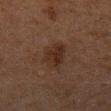No biopsy was performed on this lesion — it was imaged during a full skin examination and was not determined to be concerning.
The patient is a male aged 78–82.
The lesion is on the mid back.
Captured under cross-polarized illumination.
A roughly 15 mm field-of-view crop from a total-body skin photograph.
The total-body-photography lesion software estimated an eccentricity of roughly 0.8 and a shape-asymmetry score of about 0.25 (0 = symmetric).